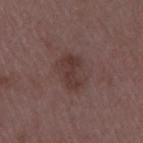No biopsy was performed on this lesion — it was imaged during a full skin examination and was not determined to be concerning.
Cropped from a whole-body photographic skin survey; the tile spans about 15 mm.
An algorithmic analysis of the crop reported a shape eccentricity near 0.8 and two-axis asymmetry of about 0.3. The analysis additionally found a lesion color around L≈35 a*≈18 b*≈19 in CIELAB and a lesion–skin lightness drop of about 7.
Approximately 4 mm at its widest.
From the right upper arm.
The tile uses white-light illumination.
The subject is a female in their mid-40s.